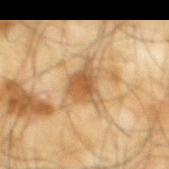Captured during whole-body skin photography for melanoma surveillance; the lesion was not biopsied.
The lesion is located on the mid back.
This image is a 15 mm lesion crop taken from a total-body photograph.
A male patient, approximately 65 years of age.